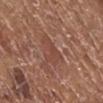Imaged during a routine full-body skin examination; the lesion was not biopsied and no histopathology is available. Approximately 3.5 mm at its widest. A 15 mm crop from a total-body photograph taken for skin-cancer surveillance. From the leg. A female patient, aged approximately 75. Captured under white-light illumination.